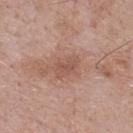Clinical impression: This lesion was catalogued during total-body skin photography and was not selected for biopsy. Clinical summary: A male subject aged 68–72. Located on the upper back. A region of skin cropped from a whole-body photographic capture, roughly 15 mm wide. This is a white-light tile.